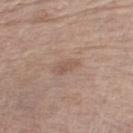This lesion was catalogued during total-body skin photography and was not selected for biopsy. The lesion is on the left lower leg. A male patient, in their 70s. A 15 mm crop from a total-body photograph taken for skin-cancer surveillance.